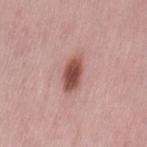Part of a total-body skin-imaging series; this lesion was reviewed on a skin check and was not flagged for biopsy. Longest diameter approximately 4.5 mm. The lesion is on the left thigh. The tile uses white-light illumination. A region of skin cropped from a whole-body photographic capture, roughly 15 mm wide. The subject is a female in their 50s.